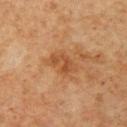{"biopsy_status": "not biopsied; imaged during a skin examination", "site": "right upper arm", "patient": {"sex": "male", "age_approx": 60}, "image": {"source": "total-body photography crop", "field_of_view_mm": 15}, "lighting": "cross-polarized"}